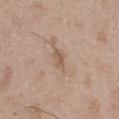Imaged during a routine full-body skin examination; the lesion was not biopsied and no histopathology is available. Automated image analysis of the tile measured an area of roughly 3.5 mm², a shape eccentricity near 0.85, and a symmetry-axis asymmetry near 0.25. The software also gave a mean CIELAB color near L≈57 a*≈16 b*≈28, about 8 CIELAB-L* units darker than the surrounding skin, and a normalized border contrast of about 6. The software also gave a border-irregularity rating of about 2.5/10, internal color variation of about 2 on a 0–10 scale, and peripheral color asymmetry of about 0.5. The analysis additionally found a nevus-likeness score of about 0/100 and a detector confidence of about 100 out of 100 that the crop contains a lesion. The tile uses white-light illumination. A roughly 15 mm field-of-view crop from a total-body skin photograph. The lesion is on the right thigh. A male patient, in their 50s.